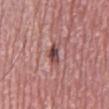Recorded during total-body skin imaging; not selected for excision or biopsy.